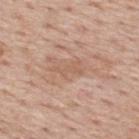Q: Is there a histopathology result?
A: catalogued during a skin exam; not biopsied
Q: Who is the patient?
A: male, aged around 75
Q: What kind of image is this?
A: ~15 mm tile from a whole-body skin photo
Q: What did automated image analysis measure?
A: a mean CIELAB color near L≈59 a*≈20 b*≈30, about 7 CIELAB-L* units darker than the surrounding skin, and a lesion-to-skin contrast of about 5 (normalized; higher = more distinct); a detector confidence of about 85 out of 100 that the crop contains a lesion
Q: Where on the body is the lesion?
A: the upper back